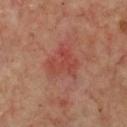biopsy status: no biopsy performed (imaged during a skin exam)
illumination: cross-polarized
automated lesion analysis: an area of roughly 8.5 mm² and an eccentricity of roughly 0.4; a mean CIELAB color near L≈43 a*≈29 b*≈27 and a normalized border contrast of about 5.5; a border-irregularity rating of about 6/10, a within-lesion color-variation index near 3.5/10, and radial color variation of about 1.5; an automated nevus-likeness rating near 0 out of 100 and a detector confidence of about 100 out of 100 that the crop contains a lesion
site: the chest
image: ~15 mm tile from a whole-body skin photo
patient: male, aged around 70